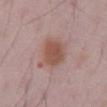patient:
  sex: male
  age_approx: 50
image:
  source: total-body photography crop
  field_of_view_mm: 15
site: abdomen